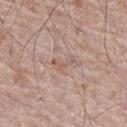Findings:
• workup · catalogued during a skin exam; not biopsied
• patient · male, approximately 70 years of age
• body site · the right thigh
• image · ~15 mm tile from a whole-body skin photo